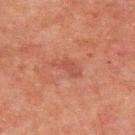The tile uses cross-polarized illumination.
A male subject, aged around 60.
From the upper back.
The lesion's longest dimension is about 4 mm.
A roughly 15 mm field-of-view crop from a total-body skin photograph.